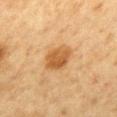Impression:
Part of a total-body skin-imaging series; this lesion was reviewed on a skin check and was not flagged for biopsy.
Acquisition and patient details:
Cropped from a total-body skin-imaging series; the visible field is about 15 mm. Approximately 4 mm at its widest. A female subject, approximately 50 years of age. The lesion is on the mid back. The lesion-visualizer software estimated an area of roughly 9 mm² and an outline eccentricity of about 0.7 (0 = round, 1 = elongated). The analysis additionally found roughly 10 lightness units darker than nearby skin. The analysis additionally found a lesion-detection confidence of about 100/100.